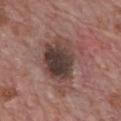<tbp_lesion>
<biopsy_status>not biopsied; imaged during a skin examination</biopsy_status>
<automated_metrics>
  <area_mm2_approx>25.0</area_mm2_approx>
  <eccentricity>0.75</eccentricity>
  <vs_skin_darker_L>12.0</vs_skin_darker_L>
  <vs_skin_contrast_norm>9.5</vs_skin_contrast_norm>
</automated_metrics>
<site>chest</site>
<image>
  <source>total-body photography crop</source>
  <field_of_view_mm>15</field_of_view_mm>
</image>
<lighting>white-light</lighting>
<lesion_size>
  <long_diameter_mm_approx>8.0</long_diameter_mm_approx>
</lesion_size>
<patient>
  <sex>male</sex>
  <age_approx>70</age_approx>
</patient>
</tbp_lesion>